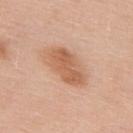Assessment:
The lesion was photographed on a routine skin check and not biopsied; there is no pathology result.
Acquisition and patient details:
Located on the upper back. A male patient aged 53–57. The total-body-photography lesion software estimated a border-irregularity rating of about 2.5/10, a color-variation rating of about 4.5/10, and radial color variation of about 1.5. The software also gave an automated nevus-likeness rating near 85 out of 100 and lesion-presence confidence of about 100/100. Captured under white-light illumination. The recorded lesion diameter is about 6 mm. Cropped from a total-body skin-imaging series; the visible field is about 15 mm.